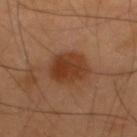The lesion was photographed on a routine skin check and not biopsied; there is no pathology result. Imaged with cross-polarized lighting. Automated image analysis of the tile measured a lesion area of about 13 mm², an outline eccentricity of about 0.4 (0 = round, 1 = elongated), and two-axis asymmetry of about 0.15. About 4 mm across. On the upper back. A 15 mm crop from a total-body photograph taken for skin-cancer surveillance. A male patient aged 38–42.